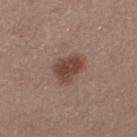The lesion is on the left thigh. A region of skin cropped from a whole-body photographic capture, roughly 15 mm wide. An algorithmic analysis of the crop reported a footprint of about 8 mm², an outline eccentricity of about 0.7 (0 = round, 1 = elongated), and two-axis asymmetry of about 0.2. It also reported a border-irregularity rating of about 2/10, a color-variation rating of about 3/10, and a peripheral color-asymmetry measure near 1. Longest diameter approximately 4 mm. The patient is a female aged around 30.